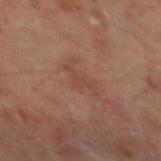Clinical impression:
The lesion was photographed on a routine skin check and not biopsied; there is no pathology result.
Clinical summary:
A male patient, aged 63 to 67. This image is a 15 mm lesion crop taken from a total-body photograph. On the mid back.